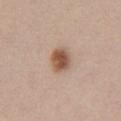| field | value |
|---|---|
| biopsy status | imaged on a skin check; not biopsied |
| location | the front of the torso |
| patient | female, aged approximately 40 |
| imaging modality | total-body-photography crop, ~15 mm field of view |